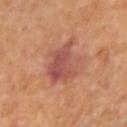– notes: imaged on a skin check; not biopsied
– subject: female, aged 38–42
– anatomic site: the right forearm
– image source: 15 mm crop, total-body photography
– lesion diameter: about 5 mm
– illumination: cross-polarized